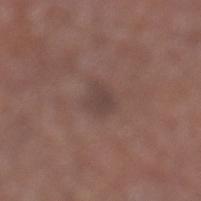Part of a total-body skin-imaging series; this lesion was reviewed on a skin check and was not flagged for biopsy.
This is a white-light tile.
A male subject in their mid-70s.
About 3 mm across.
Automated tile analysis of the lesion measured a mean CIELAB color near L≈41 a*≈16 b*≈19 and a lesion–skin lightness drop of about 6. The software also gave a color-variation rating of about 1.5/10 and peripheral color asymmetry of about 0.5.
On the left lower leg.
A 15 mm close-up extracted from a 3D total-body photography capture.